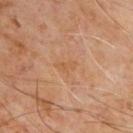Clinical impression: The lesion was tiled from a total-body skin photograph and was not biopsied. Clinical summary: From the chest. This is a cross-polarized tile. The subject is a male approximately 60 years of age. Longest diameter approximately 2.5 mm. A 15 mm close-up tile from a total-body photography series done for melanoma screening. The total-body-photography lesion software estimated an eccentricity of roughly 0.85. It also reported a nevus-likeness score of about 0/100 and lesion-presence confidence of about 100/100.